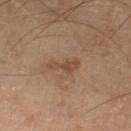notes = imaged on a skin check; not biopsied
diameter = ~3.5 mm (longest diameter)
patient = male, roughly 65 years of age
illumination = cross-polarized illumination
image = ~15 mm crop, total-body skin-cancer survey
body site = the right thigh